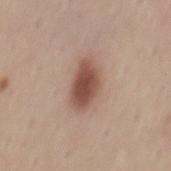Imaged during a routine full-body skin examination; the lesion was not biopsied and no histopathology is available.
This is a white-light tile.
The lesion is on the mid back.
A region of skin cropped from a whole-body photographic capture, roughly 15 mm wide.
A male patient, aged approximately 45.